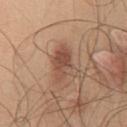Clinical impression:
The lesion was tiled from a total-body skin photograph and was not biopsied.
Image and clinical context:
This image is a 15 mm lesion crop taken from a total-body photograph. A male subject aged approximately 45. From the chest. Captured under white-light illumination. Longest diameter approximately 4.5 mm.